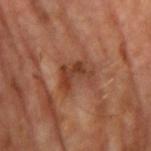Imaged during a routine full-body skin examination; the lesion was not biopsied and no histopathology is available.